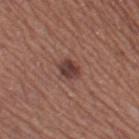This image is a 15 mm lesion crop taken from a total-body photograph.
Captured under white-light illumination.
The lesion-visualizer software estimated an eccentricity of roughly 0.7 and a shape-asymmetry score of about 0.15 (0 = symmetric). The software also gave internal color variation of about 4 on a 0–10 scale and a peripheral color-asymmetry measure near 1.5.
The lesion is on the left thigh.
A female subject, roughly 65 years of age.
Longest diameter approximately 3 mm.